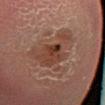This lesion was catalogued during total-body skin photography and was not selected for biopsy. The lesion's longest dimension is about 5 mm. The tile uses cross-polarized illumination. On the left lower leg. Cropped from a whole-body photographic skin survey; the tile spans about 15 mm. A male subject aged around 50.